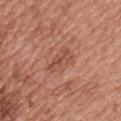The total-body-photography lesion software estimated a lesion area of about 4 mm², an outline eccentricity of about 0.95 (0 = round, 1 = elongated), and a shape-asymmetry score of about 0.4 (0 = symmetric). It also reported a lesion color around L≈50 a*≈25 b*≈29 in CIELAB, a lesion–skin lightness drop of about 8, and a normalized border contrast of about 5.5. It also reported lesion-presence confidence of about 100/100. From the upper back. The recorded lesion diameter is about 3.5 mm. A female subject, about 60 years old. A 15 mm close-up tile from a total-body photography series done for melanoma screening.